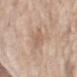Case summary:
- follow-up: total-body-photography surveillance lesion; no biopsy
- automated lesion analysis: a lesion color around L≈60 a*≈17 b*≈29 in CIELAB, a lesion–skin lightness drop of about 8, and a normalized lesion–skin contrast near 5
- patient: female, roughly 75 years of age
- site: the left forearm
- size: about 4 mm
- image: 15 mm crop, total-body photography